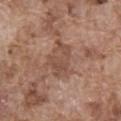workup = total-body-photography surveillance lesion; no biopsy
patient = male, aged approximately 75
image = ~15 mm tile from a whole-body skin photo
image-analysis metrics = a border-irregularity rating of about 4/10, internal color variation of about 3.5 on a 0–10 scale, and radial color variation of about 1
illumination = white-light illumination
location = the abdomen